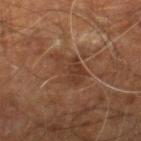<record>
  <automated_metrics>
    <area_mm2_approx>9.0</area_mm2_approx>
    <eccentricity>0.8</eccentricity>
    <shape_asymmetry>0.35</shape_asymmetry>
    <color_variation_0_10>3.0</color_variation_0_10>
    <peripheral_color_asymmetry>1.0</peripheral_color_asymmetry>
    <lesion_detection_confidence_0_100>95</lesion_detection_confidence_0_100>
  </automated_metrics>
  <image>
    <source>total-body photography crop</source>
    <field_of_view_mm>15</field_of_view_mm>
  </image>
  <site>right leg</site>
  <patient>
    <sex>male</sex>
    <age_approx>60</age_approx>
  </patient>
</record>